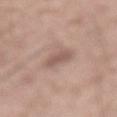The lesion was photographed on a routine skin check and not biopsied; there is no pathology result.
The lesion is on the mid back.
An algorithmic analysis of the crop reported a lesion area of about 4.5 mm², an outline eccentricity of about 0.85 (0 = round, 1 = elongated), and a symmetry-axis asymmetry near 0.25. It also reported a lesion color around L≈55 a*≈18 b*≈24 in CIELAB, roughly 9 lightness units darker than nearby skin, and a lesion-to-skin contrast of about 6.5 (normalized; higher = more distinct). And it measured a color-variation rating of about 1/10. And it measured an automated nevus-likeness rating near 5 out of 100 and a lesion-detection confidence of about 100/100.
A region of skin cropped from a whole-body photographic capture, roughly 15 mm wide.
Measured at roughly 3 mm in maximum diameter.
The subject is a male aged 58 to 62.